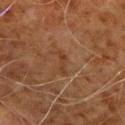{
  "biopsy_status": "not biopsied; imaged during a skin examination"
}